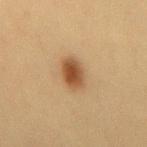The lesion was tiled from a total-body skin photograph and was not biopsied.
The tile uses cross-polarized illumination.
Cropped from a whole-body photographic skin survey; the tile spans about 15 mm.
The lesion is on the mid back.
A female patient in their 40s.
Longest diameter approximately 4 mm.